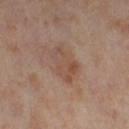Imaged during a routine full-body skin examination; the lesion was not biopsied and no histopathology is available. From the left thigh. This is a cross-polarized tile. Cropped from a whole-body photographic skin survey; the tile spans about 15 mm. The subject is a female in their mid- to late 50s.